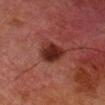Q: Was this lesion biopsied?
A: total-body-photography surveillance lesion; no biopsy
Q: What did automated image analysis measure?
A: a footprint of about 8 mm² and an eccentricity of roughly 0.35; a lesion color around L≈22 a*≈22 b*≈21 in CIELAB and a normalized border contrast of about 11
Q: Lesion location?
A: the right lower leg
Q: How was the tile lit?
A: cross-polarized
Q: How was this image acquired?
A: 15 mm crop, total-body photography
Q: Lesion size?
A: ≈3.5 mm
Q: Patient demographics?
A: male, aged around 80